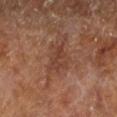Q: Was a biopsy performed?
A: no biopsy performed (imaged during a skin exam)
Q: How large is the lesion?
A: ~3.5 mm (longest diameter)
Q: Patient demographics?
A: aged approximately 65
Q: Lesion location?
A: the left lower leg
Q: How was this image acquired?
A: total-body-photography crop, ~15 mm field of view
Q: How was the tile lit?
A: cross-polarized